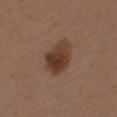{"biopsy_status": "not biopsied; imaged during a skin examination", "patient": {"sex": "female", "age_approx": 40}, "site": "left upper arm", "lighting": "white-light", "image": {"source": "total-body photography crop", "field_of_view_mm": 15}, "lesion_size": {"long_diameter_mm_approx": 4.5}}